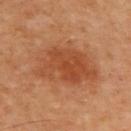The lesion was tiled from a total-body skin photograph and was not biopsied. A lesion tile, about 15 mm wide, cut from a 3D total-body photograph. A male patient, aged 48–52. Located on the upper back.